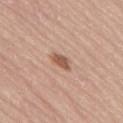{"biopsy_status": "not biopsied; imaged during a skin examination", "automated_metrics": {"nevus_likeness_0_100": 75, "lesion_detection_confidence_0_100": 100}, "image": {"source": "total-body photography crop", "field_of_view_mm": 15}, "lesion_size": {"long_diameter_mm_approx": 2.5}, "patient": {"sex": "male", "age_approx": 65}, "lighting": "white-light", "site": "lower back"}